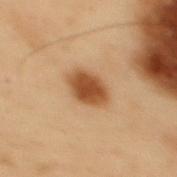The lesion was tiled from a total-body skin photograph and was not biopsied. An algorithmic analysis of the crop reported an area of roughly 8.5 mm², an outline eccentricity of about 0.55 (0 = round, 1 = elongated), and two-axis asymmetry of about 0.1. The analysis additionally found a lesion color around L≈40 a*≈19 b*≈32 in CIELAB, roughly 13 lightness units darker than nearby skin, and a normalized border contrast of about 10.5. The subject is a male aged approximately 55. The lesion is located on the mid back. A lesion tile, about 15 mm wide, cut from a 3D total-body photograph. Longest diameter approximately 3.5 mm. This is a cross-polarized tile.